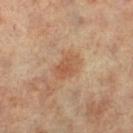The lesion was tiled from a total-body skin photograph and was not biopsied. The subject is a female roughly 65 years of age. A region of skin cropped from a whole-body photographic capture, roughly 15 mm wide. About 3.5 mm across. Located on the left leg. The tile uses cross-polarized illumination. The total-body-photography lesion software estimated a shape eccentricity near 0.75 and a shape-asymmetry score of about 0.2 (0 = symmetric). It also reported a classifier nevus-likeness of about 45/100 and a detector confidence of about 100 out of 100 that the crop contains a lesion.